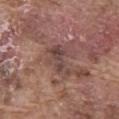No biopsy was performed on this lesion — it was imaged during a full skin examination and was not determined to be concerning.
The patient is a male in their mid-70s.
Captured under white-light illumination.
Approximately 4 mm at its widest.
Located on the abdomen.
A roughly 15 mm field-of-view crop from a total-body skin photograph.
The lesion-visualizer software estimated a lesion area of about 7 mm², an eccentricity of roughly 0.85, and a symmetry-axis asymmetry near 0.4. The analysis additionally found a mean CIELAB color near L≈43 a*≈18 b*≈21, roughly 9 lightness units darker than nearby skin, and a lesion-to-skin contrast of about 7 (normalized; higher = more distinct). The software also gave an automated nevus-likeness rating near 0 out of 100 and a detector confidence of about 70 out of 100 that the crop contains a lesion.